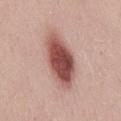size: ≈7 mm | subject: male, in their mid- to late 20s | image source: ~15 mm tile from a whole-body skin photo | anatomic site: the mid back.